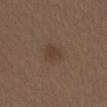follow-up = catalogued during a skin exam; not biopsied | image = total-body-photography crop, ~15 mm field of view | subject = male, aged 68–72 | automated lesion analysis = a lesion color around L≈39 a*≈15 b*≈25 in CIELAB and roughly 6 lightness units darker than nearby skin; a border-irregularity index near 2/10 and a peripheral color-asymmetry measure near 0.5 | size = about 2.5 mm | site = the lower back | tile lighting = white-light illumination.